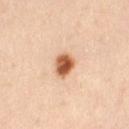A female subject approximately 40 years of age.
The lesion is located on the right thigh.
The total-body-photography lesion software estimated roughly 19 lightness units darker than nearby skin. The software also gave a detector confidence of about 100 out of 100 that the crop contains a lesion.
A roughly 15 mm field-of-view crop from a total-body skin photograph.
The tile uses cross-polarized illumination.